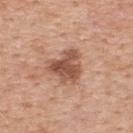Part of a total-body skin-imaging series; this lesion was reviewed on a skin check and was not flagged for biopsy.
The lesion is located on the upper back.
A female patient approximately 40 years of age.
Cropped from a whole-body photographic skin survey; the tile spans about 15 mm.
Approximately 4.5 mm at its widest.
This is a white-light tile.
The lesion-visualizer software estimated a lesion area of about 11 mm², an outline eccentricity of about 0.3 (0 = round, 1 = elongated), and a shape-asymmetry score of about 0.4 (0 = symmetric). It also reported a border-irregularity rating of about 4.5/10 and internal color variation of about 4.5 on a 0–10 scale. It also reported an automated nevus-likeness rating near 45 out of 100 and a lesion-detection confidence of about 100/100.